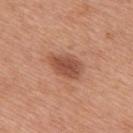{"biopsy_status": "not biopsied; imaged during a skin examination", "patient": {"sex": "male", "age_approx": 70}, "lesion_size": {"long_diameter_mm_approx": 4.5}, "image": {"source": "total-body photography crop", "field_of_view_mm": 15}, "lighting": "white-light", "site": "upper back"}